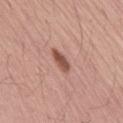biopsy status = no biopsy performed (imaged during a skin exam); acquisition = total-body-photography crop, ~15 mm field of view; patient = male, about 65 years old; anatomic site = the mid back.